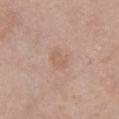workup — imaged on a skin check; not biopsied | site — the chest | patient — female, aged approximately 40 | imaging modality — ~15 mm tile from a whole-body skin photo | automated metrics — a footprint of about 4.5 mm², a shape eccentricity near 0.55, and two-axis asymmetry of about 0.25; border irregularity of about 2.5 on a 0–10 scale, internal color variation of about 1.5 on a 0–10 scale, and a peripheral color-asymmetry measure near 0.5; an automated nevus-likeness rating near 0 out of 100 | diameter — about 2.5 mm.